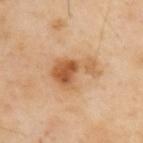- biopsy status: catalogued during a skin exam; not biopsied
- lesion diameter: ≈6 mm
- subject: male, aged 53–57
- lighting: cross-polarized
- body site: the upper back
- image source: ~15 mm tile from a whole-body skin photo